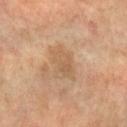The lesion was tiled from a total-body skin photograph and was not biopsied. A female subject, aged 53–57. Cropped from a total-body skin-imaging series; the visible field is about 15 mm. The lesion is on the left forearm.